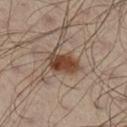Case summary:
• notes: catalogued during a skin exam; not biopsied
• lesion size: ~4 mm (longest diameter)
• illumination: cross-polarized
• subject: male, aged around 50
• image: total-body-photography crop, ~15 mm field of view
• automated metrics: a footprint of about 8.5 mm², an eccentricity of roughly 0.75, and a shape-asymmetry score of about 0.25 (0 = symmetric); a normalized border contrast of about 11; border irregularity of about 2.5 on a 0–10 scale, a color-variation rating of about 4.5/10, and peripheral color asymmetry of about 1.5; a nevus-likeness score of about 100/100
• site: the leg